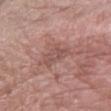Impression: Part of a total-body skin-imaging series; this lesion was reviewed on a skin check and was not flagged for biopsy. Context: A female subject aged 58–62. A close-up tile cropped from a whole-body skin photograph, about 15 mm across. Located on the left forearm. Captured under white-light illumination.